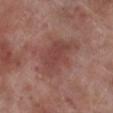Notes:
- biopsy status · no biopsy performed (imaged during a skin exam)